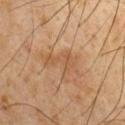  biopsy_status: not biopsied; imaged during a skin examination